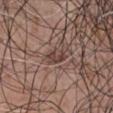Clinical impression:
Imaged during a routine full-body skin examination; the lesion was not biopsied and no histopathology is available.
Clinical summary:
A close-up tile cropped from a whole-body skin photograph, about 15 mm across. On the front of the torso. The subject is a male aged approximately 70.